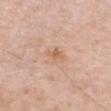<tbp_lesion>
  <lesion_size>
    <long_diameter_mm_approx>2.5</long_diameter_mm_approx>
  </lesion_size>
  <site>chest</site>
  <lighting>white-light</lighting>
  <patient>
    <sex>male</sex>
    <age_approx>70</age_approx>
  </patient>
  <image>
    <source>total-body photography crop</source>
    <field_of_view_mm>15</field_of_view_mm>
  </image>
</tbp_lesion>